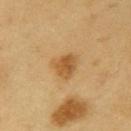No biopsy was performed on this lesion — it was imaged during a full skin examination and was not determined to be concerning. Approximately 3.5 mm at its widest. The lesion is located on the right upper arm. This is a cross-polarized tile. Cropped from a whole-body photographic skin survey; the tile spans about 15 mm. Automated tile analysis of the lesion measured border irregularity of about 2.5 on a 0–10 scale and internal color variation of about 3 on a 0–10 scale. The analysis additionally found an automated nevus-likeness rating near 95 out of 100 and lesion-presence confidence of about 100/100. A male patient, roughly 60 years of age.